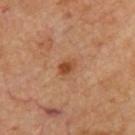<record>
  <biopsy_status>not biopsied; imaged during a skin examination</biopsy_status>
  <lesion_size>
    <long_diameter_mm_approx>2.5</long_diameter_mm_approx>
  </lesion_size>
  <site>upper back</site>
  <image>
    <source>total-body photography crop</source>
    <field_of_view_mm>15</field_of_view_mm>
  </image>
  <patient>
    <age_approx>65</age_approx>
  </patient>
  <lighting>cross-polarized</lighting>
</record>